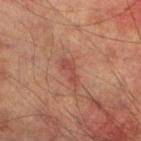Recorded during total-body skin imaging; not selected for excision or biopsy. A 15 mm close-up extracted from a 3D total-body photography capture. Captured under cross-polarized illumination. A male patient, approximately 75 years of age. On the left lower leg. About 3 mm across.